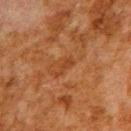| feature | finding |
|---|---|
| workup | total-body-photography surveillance lesion; no biopsy |
| imaging modality | 15 mm crop, total-body photography |
| subject | male, roughly 80 years of age |
| location | the upper back |